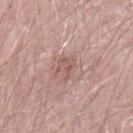| feature | finding |
|---|---|
| biopsy status | imaged on a skin check; not biopsied |
| size | about 3 mm |
| image | ~15 mm crop, total-body skin-cancer survey |
| automated lesion analysis | an area of roughly 4 mm², an outline eccentricity of about 0.65 (0 = round, 1 = elongated), and a shape-asymmetry score of about 0.5 (0 = symmetric); about 8 CIELAB-L* units darker than the surrounding skin and a lesion-to-skin contrast of about 6 (normalized; higher = more distinct); a border-irregularity index near 5.5/10, a color-variation rating of about 2.5/10, and radial color variation of about 1; a classifier nevus-likeness of about 0/100 |
| location | the left forearm |
| subject | male, aged 48 to 52 |